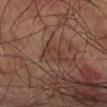Q: Is there a histopathology result?
A: total-body-photography surveillance lesion; no biopsy
Q: What lighting was used for the tile?
A: cross-polarized
Q: Lesion size?
A: ≈2.5 mm
Q: What is the anatomic site?
A: the left upper arm
Q: Automated lesion metrics?
A: an area of roughly 4 mm² and two-axis asymmetry of about 0.3
Q: What kind of image is this?
A: ~15 mm tile from a whole-body skin photo
Q: Patient demographics?
A: male, aged 73 to 77